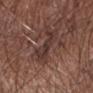<case>
<biopsy_status>not biopsied; imaged during a skin examination</biopsy_status>
<image>
  <source>total-body photography crop</source>
  <field_of_view_mm>15</field_of_view_mm>
</image>
<site>right forearm</site>
<patient>
  <sex>male</sex>
  <age_approx>70</age_approx>
</patient>
</case>